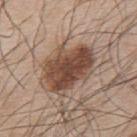Impression: Captured during whole-body skin photography for melanoma surveillance; the lesion was not biopsied. Context: The lesion is located on the back. A male subject in their mid-50s. Longest diameter approximately 6.5 mm. The lesion-visualizer software estimated a footprint of about 22 mm² and a symmetry-axis asymmetry near 0.2. The software also gave a lesion color around L≈46 a*≈18 b*≈27 in CIELAB, a lesion–skin lightness drop of about 14, and a normalized border contrast of about 10.5. A lesion tile, about 15 mm wide, cut from a 3D total-body photograph.